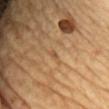Clinical summary: The tile uses cross-polarized illumination. The subject is a female in their 70s. The total-body-photography lesion software estimated an area of roughly 1 mm², an eccentricity of roughly 0.8, and a symmetry-axis asymmetry near 0.45. The software also gave a lesion color around L≈53 a*≈20 b*≈37 in CIELAB, roughly 8 lightness units darker than nearby skin, and a lesion-to-skin contrast of about 5.5 (normalized; higher = more distinct). The software also gave internal color variation of about 0 on a 0–10 scale and radial color variation of about 0. It also reported an automated nevus-likeness rating near 0 out of 100 and a lesion-detection confidence of about 70/100. The lesion's longest dimension is about 1 mm. Cropped from a total-body skin-imaging series; the visible field is about 15 mm. On the front of the torso.